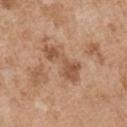The lesion was tiled from a total-body skin photograph and was not biopsied.
On the right upper arm.
A male subject, aged 53–57.
The recorded lesion diameter is about 5.5 mm.
A roughly 15 mm field-of-view crop from a total-body skin photograph.
Automated image analysis of the tile measured a nevus-likeness score of about 0/100.
Imaged with white-light lighting.